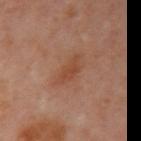The lesion was photographed on a routine skin check and not biopsied; there is no pathology result. Imaged with cross-polarized lighting. The patient is a female about 50 years old. Approximately 4.5 mm at its widest. A 15 mm crop from a total-body photograph taken for skin-cancer surveillance. From the arm.